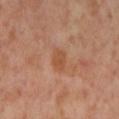The lesion was photographed on a routine skin check and not biopsied; there is no pathology result.
From the left lower leg.
The patient is a female aged 53–57.
This image is a 15 mm lesion crop taken from a total-body photograph.